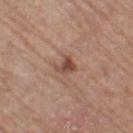Notes:
- notes · no biopsy performed (imaged during a skin exam)
- acquisition · 15 mm crop, total-body photography
- patient · female, approximately 75 years of age
- body site · the left thigh
- lesion diameter · about 2.5 mm